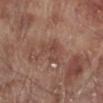Impression:
Captured during whole-body skin photography for melanoma surveillance; the lesion was not biopsied.
Clinical summary:
The lesion's longest dimension is about 4 mm. The tile uses white-light illumination. A male subject, aged around 70. Located on the right lower leg. A 15 mm close-up extracted from a 3D total-body photography capture.